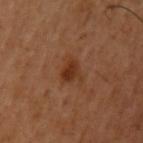{"biopsy_status": "not biopsied; imaged during a skin examination", "site": "left upper arm", "patient": {"sex": "male", "age_approx": 50}, "image": {"source": "total-body photography crop", "field_of_view_mm": 15}, "lesion_size": {"long_diameter_mm_approx": 2.5}, "lighting": "cross-polarized", "automated_metrics": {"eccentricity": 0.65, "cielab_L": 33, "cielab_a": 23, "cielab_b": 32, "vs_skin_darker_L": 9.0, "vs_skin_contrast_norm": 8.0, "border_irregularity_0_10": 3.0, "color_variation_0_10": 2.5}}